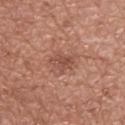Q: Was a biopsy performed?
A: total-body-photography surveillance lesion; no biopsy
Q: What is the imaging modality?
A: ~15 mm crop, total-body skin-cancer survey
Q: What is the anatomic site?
A: the back
Q: What did automated image analysis measure?
A: an area of roughly 4 mm², an eccentricity of roughly 0.75, and two-axis asymmetry of about 0.35; about 8 CIELAB-L* units darker than the surrounding skin; a border-irregularity index near 3.5/10 and a within-lesion color-variation index near 2/10
Q: Who is the patient?
A: male, aged 68–72
Q: Illumination type?
A: white-light illumination